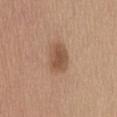{
  "biopsy_status": "not biopsied; imaged during a skin examination",
  "image": {
    "source": "total-body photography crop",
    "field_of_view_mm": 15
  },
  "patient": {
    "sex": "female",
    "age_approx": 65
  },
  "lighting": "white-light",
  "lesion_size": {
    "long_diameter_mm_approx": 3.5
  },
  "automated_metrics": {
    "border_irregularity_0_10": 1.5,
    "color_variation_0_10": 2.5,
    "peripheral_color_asymmetry": 1.0,
    "nevus_likeness_0_100": 80,
    "lesion_detection_confidence_0_100": 100
  },
  "site": "abdomen"
}